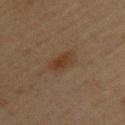image source: total-body-photography crop, ~15 mm field of view; subject: female, aged 43–47; body site: the back.